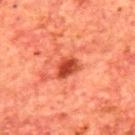workup: total-body-photography surveillance lesion; no biopsy | subject: male, in their mid-60s | size: about 3 mm | illumination: cross-polarized illumination | automated lesion analysis: an average lesion color of about L≈46 a*≈37 b*≈38 (CIELAB) and a lesion-to-skin contrast of about 9.5 (normalized; higher = more distinct); internal color variation of about 4 on a 0–10 scale and a peripheral color-asymmetry measure near 1.5 | location: the upper back | acquisition: total-body-photography crop, ~15 mm field of view.